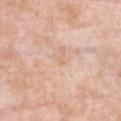  biopsy_status: not biopsied; imaged during a skin examination
  patient:
    sex: female
    age_approx: 50
  site: chest
  lesion_size:
    long_diameter_mm_approx: 1.0
  automated_metrics:
    area_mm2_approx: 1.0
    shape_asymmetry: 0.45
    cielab_L: 69
    cielab_a: 20
    cielab_b: 33
    vs_skin_darker_L: 5.0
    border_irregularity_0_10: 3.0
    peripheral_color_asymmetry: 0.0
    nevus_likeness_0_100: 0
    lesion_detection_confidence_0_100: 100
  image:
    source: total-body photography crop
    field_of_view_mm: 15